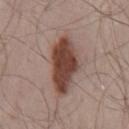An algorithmic analysis of the crop reported an area of roughly 18 mm² and a shape eccentricity near 0.9. The analysis additionally found a border-irregularity index near 2.5/10, internal color variation of about 3.5 on a 0–10 scale, and radial color variation of about 1. A region of skin cropped from a whole-body photographic capture, roughly 15 mm wide. Longest diameter approximately 7 mm. A male subject, aged approximately 50. The lesion is located on the mid back. Captured under white-light illumination.